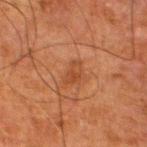The lesion was tiled from a total-body skin photograph and was not biopsied.
From the left lower leg.
A male patient, aged 78 to 82.
Cropped from a whole-body photographic skin survey; the tile spans about 15 mm.